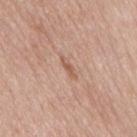No biopsy was performed on this lesion — it was imaged during a full skin examination and was not determined to be concerning. The patient is a male approximately 55 years of age. Cropped from a total-body skin-imaging series; the visible field is about 15 mm. The tile uses white-light illumination. Measured at roughly 3 mm in maximum diameter. On the mid back.